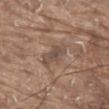This lesion was catalogued during total-body skin photography and was not selected for biopsy. This image is a 15 mm lesion crop taken from a total-body photograph. Captured under white-light illumination. The lesion-visualizer software estimated a lesion area of about 6 mm², an eccentricity of roughly 0.9, and two-axis asymmetry of about 0.25. And it measured border irregularity of about 3 on a 0–10 scale, internal color variation of about 3.5 on a 0–10 scale, and radial color variation of about 1. And it measured a nevus-likeness score of about 0/100 and lesion-presence confidence of about 55/100. From the abdomen. The patient is a male in their 80s. Longest diameter approximately 4 mm.